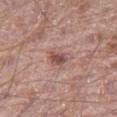A 15 mm crop from a total-body photograph taken for skin-cancer surveillance. About 2.5 mm across. The total-body-photography lesion software estimated an eccentricity of roughly 0.75 and a shape-asymmetry score of about 0.2 (0 = symmetric). It also reported a border-irregularity index near 1.5/10, a within-lesion color-variation index near 4/10, and radial color variation of about 1.5. It also reported a nevus-likeness score of about 75/100 and a lesion-detection confidence of about 100/100. A male patient, aged around 70. On the left thigh.